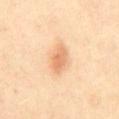This lesion was catalogued during total-body skin photography and was not selected for biopsy. The total-body-photography lesion software estimated a footprint of about 7 mm², an eccentricity of roughly 0.85, and a shape-asymmetry score of about 0.2 (0 = symmetric). The tile uses cross-polarized illumination. A female patient, aged 38 to 42. Longest diameter approximately 4 mm. The lesion is located on the back. A close-up tile cropped from a whole-body skin photograph, about 15 mm across.